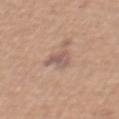<lesion>
  <biopsy_status>not biopsied; imaged during a skin examination</biopsy_status>
  <site>abdomen</site>
  <automated_metrics>
    <cielab_L>56</cielab_L>
    <cielab_a>18</cielab_a>
    <cielab_b>24</cielab_b>
    <vs_skin_darker_L>9.0</vs_skin_darker_L>
    <vs_skin_contrast_norm>7.0</vs_skin_contrast_norm>
    <color_variation_0_10>2.0</color_variation_0_10>
    <nevus_likeness_0_100>0</nevus_likeness_0_100>
  </automated_metrics>
  <lesion_size>
    <long_diameter_mm_approx>3.0</long_diameter_mm_approx>
  </lesion_size>
  <lighting>white-light</lighting>
  <patient>
    <sex>male</sex>
    <age_approx>55</age_approx>
  </patient>
  <image>
    <source>total-body photography crop</source>
    <field_of_view_mm>15</field_of_view_mm>
  </image>
</lesion>